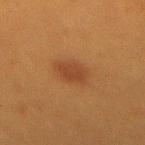biopsy status: catalogued during a skin exam; not biopsied | body site: the mid back | subject: female, aged 38–42 | image: 15 mm crop, total-body photography.